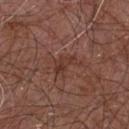Captured during whole-body skin photography for melanoma surveillance; the lesion was not biopsied. The lesion is on the chest. The lesion's longest dimension is about 3.5 mm. Imaged with white-light lighting. Automated tile analysis of the lesion measured an average lesion color of about L≈36 a*≈21 b*≈25 (CIELAB) and a normalized lesion–skin contrast near 6. It also reported border irregularity of about 4 on a 0–10 scale, a color-variation rating of about 2.5/10, and a peripheral color-asymmetry measure near 0.5. The analysis additionally found a nevus-likeness score of about 0/100 and a lesion-detection confidence of about 100/100. A male patient aged approximately 55. Cropped from a whole-body photographic skin survey; the tile spans about 15 mm.